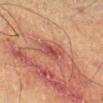Assessment: Part of a total-body skin-imaging series; this lesion was reviewed on a skin check and was not flagged for biopsy. Image and clinical context: A 15 mm close-up extracted from a 3D total-body photography capture. On the right lower leg. A male patient, aged 58–62.